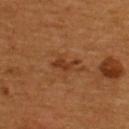Findings:
– follow-up — catalogued during a skin exam; not biopsied
– automated lesion analysis — a mean CIELAB color near L≈33 a*≈23 b*≈32 and a normalized border contrast of about 7.5; a color-variation rating of about 0/10
– acquisition — 15 mm crop, total-body photography
– location — the upper back
– patient — male, aged approximately 55
– lesion diameter — ~3 mm (longest diameter)
– lighting — cross-polarized illumination